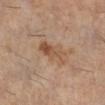Case summary:
– biopsy status: total-body-photography surveillance lesion; no biopsy
– site: the right lower leg
– subject: female, approximately 60 years of age
– automated lesion analysis: a classifier nevus-likeness of about 5/100 and lesion-presence confidence of about 100/100
– lighting: cross-polarized
– image source: ~15 mm tile from a whole-body skin photo